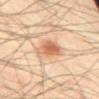Q: Was this lesion biopsied?
A: catalogued during a skin exam; not biopsied
Q: Lesion location?
A: the abdomen
Q: What is the lesion's diameter?
A: ~3.5 mm (longest diameter)
Q: Who is the patient?
A: male, aged 58–62
Q: What kind of image is this?
A: ~15 mm tile from a whole-body skin photo
Q: Illumination type?
A: cross-polarized illumination